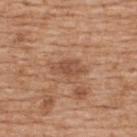Part of a total-body skin-imaging series; this lesion was reviewed on a skin check and was not flagged for biopsy. A 15 mm crop from a total-body photograph taken for skin-cancer surveillance. The patient is a male in their 60s. Captured under white-light illumination. From the upper back.